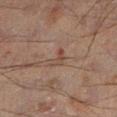This lesion was catalogued during total-body skin photography and was not selected for biopsy.
On the leg.
Longest diameter approximately 3 mm.
Cropped from a total-body skin-imaging series; the visible field is about 15 mm.
Automated tile analysis of the lesion measured an area of roughly 3 mm² and an eccentricity of roughly 0.8. And it measured an average lesion color of about L≈34 a*≈14 b*≈20 (CIELAB), roughly 5 lightness units darker than nearby skin, and a normalized lesion–skin contrast near 5.5.
A male subject, about 60 years old.
The tile uses cross-polarized illumination.